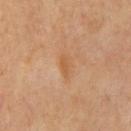Case summary:
* biopsy status: no biopsy performed (imaged during a skin exam)
* illumination: cross-polarized
* image source: ~15 mm crop, total-body skin-cancer survey
* image-analysis metrics: about 6 CIELAB-L* units darker than the surrounding skin and a lesion-to-skin contrast of about 5.5 (normalized; higher = more distinct); a border-irregularity index near 3.5/10, internal color variation of about 0 on a 0–10 scale, and a peripheral color-asymmetry measure near 0
* site: the chest
* patient: male, aged 63 to 67